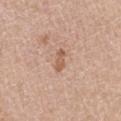Part of a total-body skin-imaging series; this lesion was reviewed on a skin check and was not flagged for biopsy. Automated image analysis of the tile measured a within-lesion color-variation index near 0.5/10 and radial color variation of about 0. The lesion is on the right thigh. A roughly 15 mm field-of-view crop from a total-body skin photograph. Approximately 3 mm at its widest. Captured under white-light illumination. A female subject aged approximately 70.